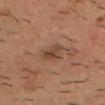biopsy status: imaged on a skin check; not biopsied
lesion diameter: ≈3.5 mm
patient: male, approximately 35 years of age
lighting: cross-polarized
automated lesion analysis: a footprint of about 7 mm², an outline eccentricity of about 0.65 (0 = round, 1 = elongated), and a symmetry-axis asymmetry near 0.2; a border-irregularity index near 2.5/10 and a within-lesion color-variation index near 4.5/10
anatomic site: the head or neck
acquisition: ~15 mm crop, total-body skin-cancer survey